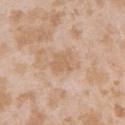{
  "biopsy_status": "not biopsied; imaged during a skin examination",
  "image": {
    "source": "total-body photography crop",
    "field_of_view_mm": 15
  },
  "patient": {
    "sex": "female",
    "age_approx": 25
  },
  "automated_metrics": {
    "eccentricity": 0.55,
    "shape_asymmetry": 0.2,
    "peripheral_color_asymmetry": 0.5,
    "nevus_likeness_0_100": 0,
    "lesion_detection_confidence_0_100": 100
  },
  "site": "left upper arm"
}